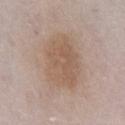Notes:
• workup — imaged on a skin check; not biopsied
• tile lighting — white-light illumination
• subject — male, aged 63 to 67
• automated lesion analysis — border irregularity of about 2 on a 0–10 scale, a within-lesion color-variation index near 3/10, and radial color variation of about 1
• imaging modality — ~15 mm crop, total-body skin-cancer survey
• diameter — ≈5.5 mm
• anatomic site — the abdomen